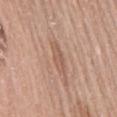<record>
  <biopsy_status>not biopsied; imaged during a skin examination</biopsy_status>
  <patient>
    <sex>female</sex>
    <age_approx>60</age_approx>
  </patient>
  <image>
    <source>total-body photography crop</source>
    <field_of_view_mm>15</field_of_view_mm>
  </image>
  <lesion_size>
    <long_diameter_mm_approx>4.5</long_diameter_mm_approx>
  </lesion_size>
  <site>mid back</site>
  <lighting>white-light</lighting>
</record>